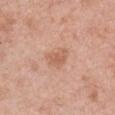Q: What kind of image is this?
A: 15 mm crop, total-body photography
Q: Who is the patient?
A: female, approximately 60 years of age
Q: Where on the body is the lesion?
A: the chest